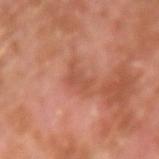{"biopsy_status": "not biopsied; imaged during a skin examination", "site": "left forearm", "lighting": "cross-polarized", "image": {"source": "total-body photography crop", "field_of_view_mm": 15}, "automated_metrics": {"area_mm2_approx": 6.0, "eccentricity": 0.85, "shape_asymmetry": 0.4, "cielab_L": 51, "cielab_a": 26, "cielab_b": 31, "vs_skin_darker_L": 7.0, "border_irregularity_0_10": 5.0, "color_variation_0_10": 2.0, "peripheral_color_asymmetry": 0.5}, "patient": {"sex": "male", "age_approx": 30}, "lesion_size": {"long_diameter_mm_approx": 4.0}}